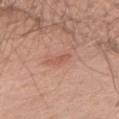tile lighting: white-light illumination; body site: the left upper arm; lesion diameter: ~2.5 mm (longest diameter); patient: male, approximately 30 years of age; image: ~15 mm crop, total-body skin-cancer survey.